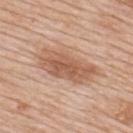Q: What is the lesion's diameter?
A: ≈7.5 mm
Q: Who is the patient?
A: male, roughly 60 years of age
Q: What did automated image analysis measure?
A: a symmetry-axis asymmetry near 0.15; an average lesion color of about L≈58 a*≈21 b*≈32 (CIELAB), a lesion–skin lightness drop of about 11, and a normalized border contrast of about 7.5; a border-irregularity rating of about 3/10, a color-variation rating of about 4/10, and radial color variation of about 1.5; lesion-presence confidence of about 100/100
Q: What lighting was used for the tile?
A: white-light illumination
Q: What is the imaging modality?
A: total-body-photography crop, ~15 mm field of view
Q: Where on the body is the lesion?
A: the upper back